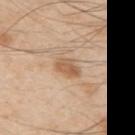lighting — white-light illumination | TBP lesion metrics — a peripheral color-asymmetry measure near 1; lesion-presence confidence of about 100/100 | site — the right upper arm | subject — male, aged 48–52 | lesion diameter — ~2.5 mm (longest diameter) | image — total-body-photography crop, ~15 mm field of view.